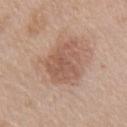Q: Was a biopsy performed?
A: total-body-photography surveillance lesion; no biopsy
Q: Patient demographics?
A: female, aged 73 to 77
Q: Lesion location?
A: the chest
Q: How was this image acquired?
A: ~15 mm tile from a whole-body skin photo
Q: What is the lesion's diameter?
A: ~5.5 mm (longest diameter)